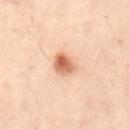Clinical impression: This lesion was catalogued during total-body skin photography and was not selected for biopsy. Image and clinical context: On the front of the torso. A 15 mm close-up tile from a total-body photography series done for melanoma screening. A female patient, about 30 years old.